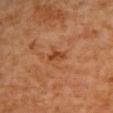Case summary:
– biopsy status: catalogued during a skin exam; not biopsied
– lesion diameter: ≈2.5 mm
– image: total-body-photography crop, ~15 mm field of view
– illumination: cross-polarized illumination
– location: the upper back
– patient: female